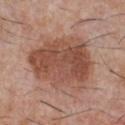No biopsy was performed on this lesion — it was imaged during a full skin examination and was not determined to be concerning.
A 15 mm close-up tile from a total-body photography series done for melanoma screening.
The subject is a male approximately 30 years of age.
About 8 mm across.
The lesion is on the chest.
This is a white-light tile.
The total-body-photography lesion software estimated an eccentricity of roughly 0.6 and a symmetry-axis asymmetry near 0.2. And it measured a border-irregularity index near 2.5/10 and radial color variation of about 2.